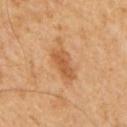biopsy status: no biopsy performed (imaged during a skin exam) | subject: male, roughly 65 years of age | location: the mid back | acquisition: ~15 mm crop, total-body skin-cancer survey | automated lesion analysis: an area of roughly 7 mm², an eccentricity of roughly 0.9, and a shape-asymmetry score of about 0.3 (0 = symmetric); a border-irregularity index near 3/10, internal color variation of about 3 on a 0–10 scale, and radial color variation of about 1 | tile lighting: cross-polarized illumination | size: ≈4.5 mm.